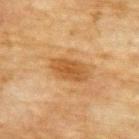automated metrics — a footprint of about 8.5 mm², a shape eccentricity near 0.85, and a shape-asymmetry score of about 0.15 (0 = symmetric); a color-variation rating of about 2.5/10; an automated nevus-likeness rating near 90 out of 100 and a detector confidence of about 100 out of 100 that the crop contains a lesion
tile lighting — cross-polarized
patient — female, approximately 80 years of age
anatomic site — the back
acquisition — total-body-photography crop, ~15 mm field of view